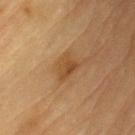workup: total-body-photography surveillance lesion; no biopsy | acquisition: total-body-photography crop, ~15 mm field of view | site: the left upper arm | subject: male, in their mid-80s or older.